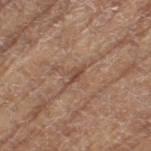Clinical impression:
The lesion was tiled from a total-body skin photograph and was not biopsied.
Image and clinical context:
On the leg. Cropped from a total-body skin-imaging series; the visible field is about 15 mm. Longest diameter approximately 3 mm. This is a white-light tile. The subject is a female in their mid- to late 70s.